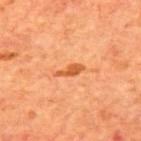The patient is a male aged 68 to 72. Approximately 3.5 mm at its widest. A 15 mm close-up tile from a total-body photography series done for melanoma screening. Located on the upper back. Imaged with cross-polarized lighting. Automated image analysis of the tile measured an average lesion color of about L≈58 a*≈32 b*≈46 (CIELAB), about 11 CIELAB-L* units darker than the surrounding skin, and a lesion-to-skin contrast of about 8 (normalized; higher = more distinct). It also reported border irregularity of about 3.5 on a 0–10 scale, internal color variation of about 0.5 on a 0–10 scale, and a peripheral color-asymmetry measure near 0. The software also gave an automated nevus-likeness rating near 30 out of 100 and lesion-presence confidence of about 100/100.